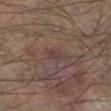follow-up: total-body-photography surveillance lesion; no biopsy
image-analysis metrics: a shape eccentricity near 0.85 and two-axis asymmetry of about 0.35; a lesion–skin lightness drop of about 5 and a normalized lesion–skin contrast near 4.5
tile lighting: cross-polarized
imaging modality: ~15 mm tile from a whole-body skin photo
location: the right lower leg
subject: male, aged around 55